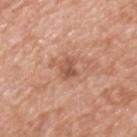Assessment: No biopsy was performed on this lesion — it was imaged during a full skin examination and was not determined to be concerning. Clinical summary: The patient is a male aged around 50. A lesion tile, about 15 mm wide, cut from a 3D total-body photograph. Approximately 2.5 mm at its widest. The lesion is located on the upper back.